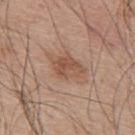Q: Was this lesion biopsied?
A: imaged on a skin check; not biopsied
Q: What is the lesion's diameter?
A: ~3.5 mm (longest diameter)
Q: What are the patient's age and sex?
A: male, aged 53–57
Q: What is the anatomic site?
A: the upper back
Q: What kind of image is this?
A: total-body-photography crop, ~15 mm field of view
Q: Automated lesion metrics?
A: an area of roughly 7 mm², a shape eccentricity near 0.7, and a symmetry-axis asymmetry near 0.25; an average lesion color of about L≈52 a*≈20 b*≈29 (CIELAB), a lesion–skin lightness drop of about 9, and a normalized lesion–skin contrast near 6.5